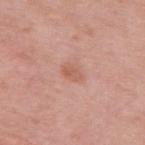{"biopsy_status": "not biopsied; imaged during a skin examination", "site": "upper back", "lesion_size": {"long_diameter_mm_approx": 2.5}, "image": {"source": "total-body photography crop", "field_of_view_mm": 15}, "patient": {"sex": "female", "age_approx": 60}, "lighting": "white-light"}